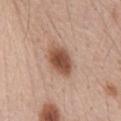Clinical impression:
Captured during whole-body skin photography for melanoma surveillance; the lesion was not biopsied.
Acquisition and patient details:
The lesion is on the abdomen. The lesion's longest dimension is about 5 mm. A male subject aged approximately 60. This is a white-light tile. An algorithmic analysis of the crop reported an area of roughly 11 mm² and two-axis asymmetry of about 0.15. The analysis additionally found a within-lesion color-variation index near 5/10 and a peripheral color-asymmetry measure near 1.5. And it measured a nevus-likeness score of about 100/100 and a detector confidence of about 100 out of 100 that the crop contains a lesion. A roughly 15 mm field-of-view crop from a total-body skin photograph.